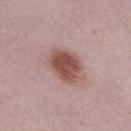Case summary:
* image source · total-body-photography crop, ~15 mm field of view
* patient · female, aged 38 to 42
* illumination · white-light
* body site · the right lower leg